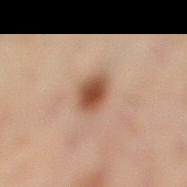{"biopsy_status": "not biopsied; imaged during a skin examination", "automated_metrics": {"border_irregularity_0_10": 1.5, "color_variation_0_10": 4.0, "peripheral_color_asymmetry": 1.0, "nevus_likeness_0_100": 100, "lesion_detection_confidence_0_100": 100}, "lighting": "cross-polarized", "patient": {"sex": "female", "age_approx": 55}, "image": {"source": "total-body photography crop", "field_of_view_mm": 15}, "lesion_size": {"long_diameter_mm_approx": 3.0}, "site": "left leg"}